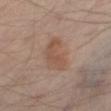Impression:
Recorded during total-body skin imaging; not selected for excision or biopsy.
Acquisition and patient details:
Captured under cross-polarized illumination. The lesion is on the right thigh. Automated tile analysis of the lesion measured an average lesion color of about L≈51 a*≈17 b*≈27 (CIELAB), a lesion–skin lightness drop of about 6, and a lesion-to-skin contrast of about 5.5 (normalized; higher = more distinct). Approximately 4.5 mm at its widest. The patient is aged around 55. A lesion tile, about 15 mm wide, cut from a 3D total-body photograph.